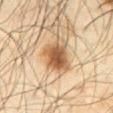{
  "biopsy_status": "not biopsied; imaged during a skin examination",
  "lighting": "cross-polarized",
  "patient": {
    "sex": "male",
    "age_approx": 65
  },
  "image": {
    "source": "total-body photography crop",
    "field_of_view_mm": 15
  },
  "site": "abdomen",
  "lesion_size": {
    "long_diameter_mm_approx": 3.5
  },
  "automated_metrics": {
    "eccentricity": 0.45,
    "shape_asymmetry": 0.15,
    "border_irregularity_0_10": 2.0,
    "color_variation_0_10": 5.0,
    "peripheral_color_asymmetry": 1.5
  }
}